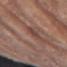The lesion was photographed on a routine skin check and not biopsied; there is no pathology result. The lesion is located on the right forearm. A female subject aged approximately 75. The recorded lesion diameter is about 3 mm. A region of skin cropped from a whole-body photographic capture, roughly 15 mm wide. The tile uses white-light illumination.